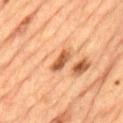Impression: Part of a total-body skin-imaging series; this lesion was reviewed on a skin check and was not flagged for biopsy. Background: A 15 mm close-up extracted from a 3D total-body photography capture. On the lower back. A male subject, roughly 85 years of age or older.